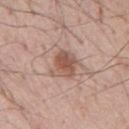  biopsy_status: not biopsied; imaged during a skin examination
  automated_metrics:
    cielab_L: 55
    cielab_a: 19
    cielab_b: 27
    vs_skin_darker_L: 11.0
    vs_skin_contrast_norm: 7.5
    nevus_likeness_0_100: 90
    lesion_detection_confidence_0_100: 100
  image:
    source: total-body photography crop
    field_of_view_mm: 15
  lesion_size:
    long_diameter_mm_approx: 3.5
  site: chest
  patient:
    sex: male
    age_approx: 55